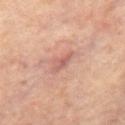• workup: no biopsy performed (imaged during a skin exam)
• lesion diameter: ≈2.5 mm
• patient: male, aged around 65
• site: the back
• automated lesion analysis: a shape eccentricity near 0.9
• acquisition: 15 mm crop, total-body photography
• illumination: cross-polarized